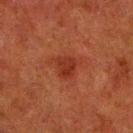notes: total-body-photography surveillance lesion; no biopsy | lesion diameter: ~2.5 mm (longest diameter) | image source: ~15 mm crop, total-body skin-cancer survey | illumination: cross-polarized illumination | subject: male, roughly 80 years of age | body site: the right lower leg.